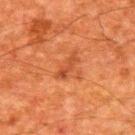Case summary:
• follow-up: imaged on a skin check; not biopsied
• site: the upper back
• lighting: cross-polarized illumination
• diameter: about 3 mm
• imaging modality: 15 mm crop, total-body photography
• subject: male, aged 58 to 62